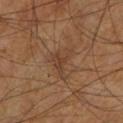This lesion was catalogued during total-body skin photography and was not selected for biopsy. A 15 mm close-up tile from a total-body photography series done for melanoma screening. About 4 mm across. The subject is a male approximately 65 years of age. This is a cross-polarized tile. Automated tile analysis of the lesion measured a footprint of about 5.5 mm² and a shape-asymmetry score of about 0.65 (0 = symmetric). Located on the left lower leg.